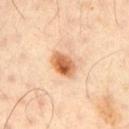Case summary:
- biopsy status · imaged on a skin check; not biopsied